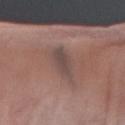{
  "site": "arm",
  "patient": {
    "sex": "male",
    "age_approx": 70
  },
  "image": {
    "source": "total-body photography crop",
    "field_of_view_mm": 15
  },
  "lesion_size": {
    "long_diameter_mm_approx": 4.0
  },
  "lighting": "white-light",
  "automated_metrics": {
    "area_mm2_approx": 7.5,
    "eccentricity": 0.8,
    "shape_asymmetry": 0.25,
    "cielab_L": 46,
    "cielab_a": 16,
    "cielab_b": 19,
    "vs_skin_darker_L": 8.0,
    "vs_skin_contrast_norm": 7.0,
    "border_irregularity_0_10": 3.0,
    "color_variation_0_10": 2.5,
    "peripheral_color_asymmetry": 1.0,
    "nevus_likeness_0_100": 0,
    "lesion_detection_confidence_0_100": 60
  }
}